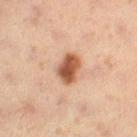The lesion is on the right thigh. A female patient aged 38 to 42. Imaged with cross-polarized lighting. The total-body-photography lesion software estimated about 17 CIELAB-L* units darker than the surrounding skin. The software also gave a classifier nevus-likeness of about 100/100 and lesion-presence confidence of about 100/100. A lesion tile, about 15 mm wide, cut from a 3D total-body photograph.